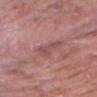Imaged during a routine full-body skin examination; the lesion was not biopsied and no histopathology is available. Imaged with white-light lighting. A male patient, approximately 70 years of age. A region of skin cropped from a whole-body photographic capture, roughly 15 mm wide. Approximately 3.5 mm at its widest. The lesion is on the chest.